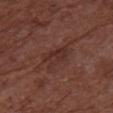Recorded during total-body skin imaging; not selected for excision or biopsy.
The lesion is located on the chest.
A female subject, roughly 80 years of age.
A 15 mm crop from a total-body photograph taken for skin-cancer surveillance.
Captured under white-light illumination.